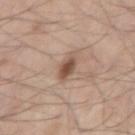Case summary:
- notes: catalogued during a skin exam; not biopsied
- image: total-body-photography crop, ~15 mm field of view
- tile lighting: white-light illumination
- patient: male, aged 68–72
- lesion size: about 2.5 mm
- anatomic site: the arm
- automated metrics: a footprint of about 4.5 mm², an outline eccentricity of about 0.65 (0 = round, 1 = elongated), and a shape-asymmetry score of about 0.25 (0 = symmetric); a mean CIELAB color near L≈51 a*≈18 b*≈27, about 12 CIELAB-L* units darker than the surrounding skin, and a lesion-to-skin contrast of about 8.5 (normalized; higher = more distinct); a nevus-likeness score of about 90/100 and lesion-presence confidence of about 100/100